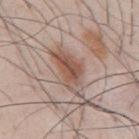The lesion was photographed on a routine skin check and not biopsied; there is no pathology result.
A 15 mm close-up tile from a total-body photography series done for melanoma screening.
Captured under white-light illumination.
A male patient about 35 years old.
Approximately 6 mm at its widest.
The lesion is located on the mid back.